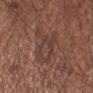The tile uses white-light illumination. From the arm. A male patient, in their mid- to late 50s. Longest diameter approximately 4.5 mm. A roughly 15 mm field-of-view crop from a total-body skin photograph.